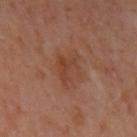notes: no biopsy performed (imaged during a skin exam); acquisition: ~15 mm tile from a whole-body skin photo; subject: female, about 50 years old; illumination: cross-polarized illumination; body site: the right upper arm.